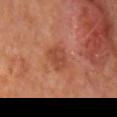No biopsy was performed on this lesion — it was imaged during a full skin examination and was not determined to be concerning. Captured under cross-polarized illumination. The subject is a male roughly 65 years of age. A lesion tile, about 15 mm wide, cut from a 3D total-body photograph. From the mid back.